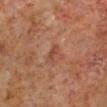| field | value |
|---|---|
| notes | imaged on a skin check; not biopsied |
| image-analysis metrics | an area of roughly 2.5 mm² and a shape eccentricity near 0.9; an average lesion color of about L≈43 a*≈25 b*≈29 (CIELAB), roughly 7 lightness units darker than nearby skin, and a lesion-to-skin contrast of about 6 (normalized; higher = more distinct) |
| illumination | cross-polarized |
| patient | male, aged approximately 60 |
| anatomic site | the leg |
| size | ~2.5 mm (longest diameter) |
| imaging modality | total-body-photography crop, ~15 mm field of view |